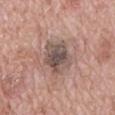  patient:
    sex: male
    age_approx: 70
  lighting: white-light
  image:
    source: total-body photography crop
    field_of_view_mm: 15
  site: mid back
  lesion_size:
    long_diameter_mm_approx: 5.5